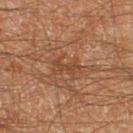{"patient": {"sex": "male", "age_approx": 60}, "site": "leg", "image": {"source": "total-body photography crop", "field_of_view_mm": 15}}